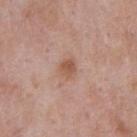follow-up=catalogued during a skin exam; not biopsied | acquisition=~15 mm tile from a whole-body skin photo | body site=the chest | patient=male, in their mid- to late 50s | tile lighting=white-light illumination | lesion size=~2.5 mm (longest diameter).